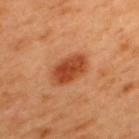The lesion was tiled from a total-body skin photograph and was not biopsied.
Located on the back.
The subject is a male in their 50s.
A region of skin cropped from a whole-body photographic capture, roughly 15 mm wide.